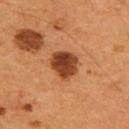Impression: Recorded during total-body skin imaging; not selected for excision or biopsy. Context: The lesion-visualizer software estimated a footprint of about 9.5 mm² and a shape-asymmetry score of about 0.2 (0 = symmetric). The analysis additionally found an average lesion color of about L≈39 a*≈25 b*≈35 (CIELAB) and a normalized border contrast of about 12. And it measured border irregularity of about 2 on a 0–10 scale, a within-lesion color-variation index near 4.5/10, and peripheral color asymmetry of about 1.5. And it measured a lesion-detection confidence of about 100/100. A 15 mm close-up tile from a total-body photography series done for melanoma screening. Imaged with cross-polarized lighting. From the upper back. Approximately 3.5 mm at its widest. A male patient in their mid- to late 50s.